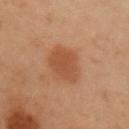Notes:
- notes: total-body-photography surveillance lesion; no biopsy
- lesion size: ~4.5 mm (longest diameter)
- tile lighting: cross-polarized illumination
- patient: male, about 55 years old
- body site: the arm
- acquisition: ~15 mm tile from a whole-body skin photo